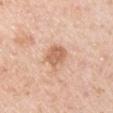{
  "biopsy_status": "not biopsied; imaged during a skin examination",
  "lighting": "white-light",
  "site": "arm",
  "lesion_size": {
    "long_diameter_mm_approx": 3.0
  },
  "image": {
    "source": "total-body photography crop",
    "field_of_view_mm": 15
  },
  "patient": {
    "sex": "male",
    "age_approx": 40
  }
}